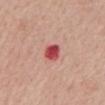Notes:
* biopsy status: imaged on a skin check; not biopsied
* subject: male, aged approximately 60
* lesion diameter: ~3 mm (longest diameter)
* image-analysis metrics: border irregularity of about 1.5 on a 0–10 scale and peripheral color asymmetry of about 1
* acquisition: ~15 mm crop, total-body skin-cancer survey
* site: the mid back